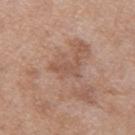Findings:
- biopsy status — catalogued during a skin exam; not biopsied